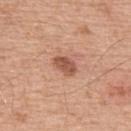Notes:
– notes · total-body-photography surveillance lesion; no biopsy
– lesion size · ≈3 mm
– imaging modality · total-body-photography crop, ~15 mm field of view
– subject · male, aged 53–57
– anatomic site · the upper back